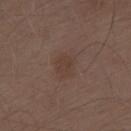- notes · catalogued during a skin exam; not biopsied
- size · ~3 mm (longest diameter)
- tile lighting · white-light illumination
- location · the lower back
- image · ~15 mm crop, total-body skin-cancer survey
- subject · male, roughly 70 years of age